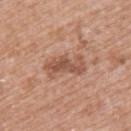notes: total-body-photography surveillance lesion; no biopsy
image-analysis metrics: a lesion area of about 9 mm² and a shape eccentricity near 0.9; a border-irregularity index near 5/10, a within-lesion color-variation index near 3.5/10, and a peripheral color-asymmetry measure near 1; lesion-presence confidence of about 100/100
subject: female, aged 38–42
body site: the upper back
tile lighting: white-light
size: ≈5 mm
image source: ~15 mm tile from a whole-body skin photo